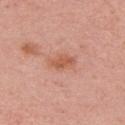{
  "biopsy_status": "not biopsied; imaged during a skin examination",
  "image": {
    "source": "total-body photography crop",
    "field_of_view_mm": 15
  },
  "site": "right upper arm",
  "lighting": "white-light",
  "patient": {
    "sex": "female",
    "age_approx": 35
  },
  "lesion_size": {
    "long_diameter_mm_approx": 3.5
  },
  "automated_metrics": {
    "area_mm2_approx": 5.5,
    "eccentricity": 0.8,
    "shape_asymmetry": 0.25,
    "cielab_L": 58,
    "cielab_a": 26,
    "cielab_b": 33,
    "vs_skin_darker_L": 9.0,
    "border_irregularity_0_10": 3.0,
    "color_variation_0_10": 2.0,
    "nevus_likeness_0_100": 0,
    "lesion_detection_confidence_0_100": 100
  }
}